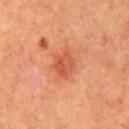Q: Is there a histopathology result?
A: imaged on a skin check; not biopsied
Q: Lesion size?
A: about 3.5 mm
Q: Who is the patient?
A: male, approximately 65 years of age
Q: Illumination type?
A: cross-polarized
Q: What is the imaging modality?
A: total-body-photography crop, ~15 mm field of view
Q: Where on the body is the lesion?
A: the chest